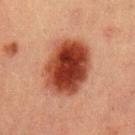• notes · catalogued during a skin exam; not biopsied
• acquisition · ~15 mm crop, total-body skin-cancer survey
• patient · male, roughly 40 years of age
• location · the left upper arm